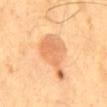Part of a total-body skin-imaging series; this lesion was reviewed on a skin check and was not flagged for biopsy. From the mid back. A male subject, about 60 years old. The lesion-visualizer software estimated an average lesion color of about L≈68 a*≈25 b*≈42 (CIELAB), roughly 11 lightness units darker than nearby skin, and a lesion-to-skin contrast of about 6.5 (normalized; higher = more distinct). And it measured an automated nevus-likeness rating near 85 out of 100 and a detector confidence of about 100 out of 100 that the crop contains a lesion. Cropped from a total-body skin-imaging series; the visible field is about 15 mm. This is a cross-polarized tile.